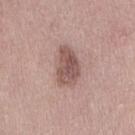Captured during whole-body skin photography for melanoma surveillance; the lesion was not biopsied.
Automated image analysis of the tile measured a border-irregularity index near 3/10 and a color-variation rating of about 4/10. The software also gave an automated nevus-likeness rating near 25 out of 100.
The patient is a female roughly 40 years of age.
This is a white-light tile.
On the right thigh.
The recorded lesion diameter is about 5 mm.
This image is a 15 mm lesion crop taken from a total-body photograph.